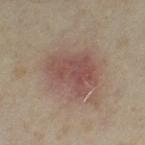Clinical summary:
The total-body-photography lesion software estimated an average lesion color of about L≈47 a*≈18 b*≈21 (CIELAB), about 8 CIELAB-L* units darker than the surrounding skin, and a lesion-to-skin contrast of about 6.5 (normalized; higher = more distinct). The lesion is on the left thigh. Cropped from a whole-body photographic skin survey; the tile spans about 15 mm. A female subject about 35 years old.